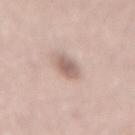{"biopsy_status": "not biopsied; imaged during a skin examination", "lesion_size": {"long_diameter_mm_approx": 3.0}, "lighting": "white-light", "site": "lower back", "patient": {"sex": "female", "age_approx": 65}, "image": {"source": "total-body photography crop", "field_of_view_mm": 15}}